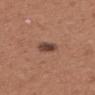Q: Lesion size?
A: ~3 mm (longest diameter)
Q: What is the anatomic site?
A: the upper back
Q: How was the tile lit?
A: white-light
Q: Patient demographics?
A: female, aged 63 to 67
Q: What kind of image is this?
A: ~15 mm crop, total-body skin-cancer survey